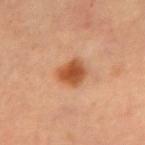Imaged during a routine full-body skin examination; the lesion was not biopsied and no histopathology is available.
A female subject, approximately 60 years of age.
From the left forearm.
A 15 mm close-up tile from a total-body photography series done for melanoma screening.